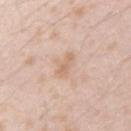Recorded during total-body skin imaging; not selected for excision or biopsy. Longest diameter approximately 3.5 mm. A 15 mm close-up tile from a total-body photography series done for melanoma screening. This is a white-light tile. The lesion is on the arm. An algorithmic analysis of the crop reported a footprint of about 3.5 mm² and an outline eccentricity of about 0.95 (0 = round, 1 = elongated). And it measured border irregularity of about 4 on a 0–10 scale and radial color variation of about 0. A male patient aged approximately 25.